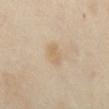Assessment: Part of a total-body skin-imaging series; this lesion was reviewed on a skin check and was not flagged for biopsy. Image and clinical context: The recorded lesion diameter is about 3 mm. The lesion is located on the abdomen. This is a cross-polarized tile. A female subject aged 53 to 57. The lesion-visualizer software estimated about 6 CIELAB-L* units darker than the surrounding skin. It also reported a classifier nevus-likeness of about 10/100 and lesion-presence confidence of about 100/100. A 15 mm crop from a total-body photograph taken for skin-cancer surveillance.